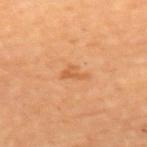Impression: Recorded during total-body skin imaging; not selected for excision or biopsy. Image and clinical context: The patient is a female aged 68 to 72. A close-up tile cropped from a whole-body skin photograph, about 15 mm across. Located on the upper back.